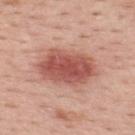Case summary:
- workup: imaged on a skin check; not biopsied
- body site: the upper back
- subject: male, aged 63 to 67
- acquisition: 15 mm crop, total-body photography